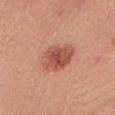On the head or neck.
The lesion-visualizer software estimated an area of roughly 12 mm², an eccentricity of roughly 0.65, and a shape-asymmetry score of about 0.1 (0 = symmetric). The analysis additionally found an average lesion color of about L≈53 a*≈28 b*≈31 (CIELAB) and a normalized border contrast of about 8. The analysis additionally found border irregularity of about 1.5 on a 0–10 scale and internal color variation of about 4.5 on a 0–10 scale.
A close-up tile cropped from a whole-body skin photograph, about 15 mm across.
The subject is a female about 45 years old.
The recorded lesion diameter is about 4.5 mm.
This is a white-light tile.